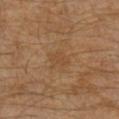This lesion was catalogued during total-body skin photography and was not selected for biopsy. From the left leg. About 2.5 mm across. The subject is a male aged around 30. A 15 mm close-up extracted from a 3D total-body photography capture. An algorithmic analysis of the crop reported a lesion area of about 3.5 mm² and an eccentricity of roughly 0.75.